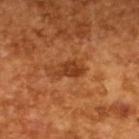Part of a total-body skin-imaging series; this lesion was reviewed on a skin check and was not flagged for biopsy. The subject is a male aged around 65. A roughly 15 mm field-of-view crop from a total-body skin photograph. The lesion's longest dimension is about 3 mm. Captured under cross-polarized illumination.